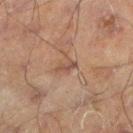- follow-up · catalogued during a skin exam; not biopsied
- image · ~15 mm crop, total-body skin-cancer survey
- lighting · cross-polarized illumination
- patient · male, aged 58 to 62
- body site · the left lower leg